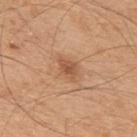{"patient": {"sex": "male", "age_approx": 50}, "lighting": "white-light", "automated_metrics": {"area_mm2_approx": 4.0, "eccentricity": 0.75, "shape_asymmetry": 0.3}, "image": {"source": "total-body photography crop", "field_of_view_mm": 15}, "site": "back", "lesion_size": {"long_diameter_mm_approx": 3.0}}